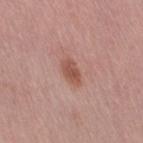– workup — catalogued during a skin exam; not biopsied
– image — ~15 mm crop, total-body skin-cancer survey
– site — the leg
– tile lighting — white-light illumination
– patient — female, approximately 40 years of age